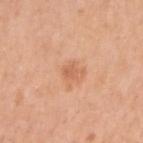Case summary:
- tile lighting — white-light illumination
- TBP lesion metrics — a footprint of about 3 mm² and a shape eccentricity near 0.8; an average lesion color of about L≈62 a*≈25 b*≈36 (CIELAB) and a normalized border contrast of about 5.5; a border-irregularity rating of about 4/10 and a color-variation rating of about 1/10
- diameter — ~2.5 mm (longest diameter)
- anatomic site — the right upper arm
- image source — ~15 mm tile from a whole-body skin photo
- patient — female, approximately 50 years of age